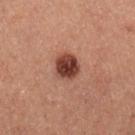Findings:
– notes · imaged on a skin check; not biopsied
– image · 15 mm crop, total-body photography
– location · the right lower leg
– lighting · cross-polarized
– subject · male, approximately 60 years of age